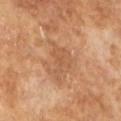Impression: The lesion was tiled from a total-body skin photograph and was not biopsied. Acquisition and patient details: Cropped from a whole-body photographic skin survey; the tile spans about 15 mm. An algorithmic analysis of the crop reported an area of roughly 8 mm² and a symmetry-axis asymmetry near 0.5. The analysis additionally found radial color variation of about 0.5. This is a cross-polarized tile. A male patient roughly 65 years of age. Longest diameter approximately 4.5 mm.